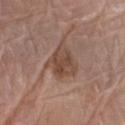Notes:
– biopsy status — no biopsy performed (imaged during a skin exam)
– site — the right forearm
– image — ~15 mm crop, total-body skin-cancer survey
– lesion size — ~4 mm (longest diameter)
– subject — male, aged around 80
– TBP lesion metrics — a border-irregularity index near 3/10 and internal color variation of about 4 on a 0–10 scale